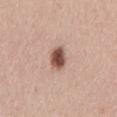This lesion was catalogued during total-body skin photography and was not selected for biopsy. The lesion's longest dimension is about 2.5 mm. On the back. Imaged with white-light lighting. A lesion tile, about 15 mm wide, cut from a 3D total-body photograph. A female patient, roughly 30 years of age.